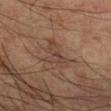biopsy status = no biopsy performed (imaged during a skin exam)
acquisition = ~15 mm crop, total-body skin-cancer survey
location = the right forearm
patient = male, about 60 years old